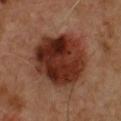This lesion was catalogued during total-body skin photography and was not selected for biopsy. A male subject, about 50 years old. Cropped from a whole-body photographic skin survey; the tile spans about 15 mm. Captured under cross-polarized illumination. From the chest.